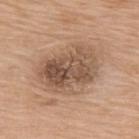Impression:
The lesion was tiled from a total-body skin photograph and was not biopsied.
Clinical summary:
Longest diameter approximately 7.5 mm. A close-up tile cropped from a whole-body skin photograph, about 15 mm across. The lesion is on the back. A female patient in their mid-70s. Captured under white-light illumination.